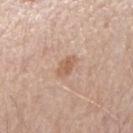No biopsy was performed on this lesion — it was imaged during a full skin examination and was not determined to be concerning. A male subject, approximately 60 years of age. Captured under white-light illumination. Measured at roughly 2.5 mm in maximum diameter. A 15 mm close-up extracted from a 3D total-body photography capture. The lesion is located on the right forearm.